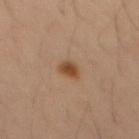The lesion was photographed on a routine skin check and not biopsied; there is no pathology result. About 2.5 mm across. Located on the chest. Cropped from a total-body skin-imaging series; the visible field is about 15 mm. This is a cross-polarized tile. A male patient in their mid- to late 30s.